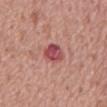| feature | finding |
|---|---|
| follow-up | imaged on a skin check; not biopsied |
| diameter | about 3 mm |
| image source | ~15 mm tile from a whole-body skin photo |
| body site | the back |
| image-analysis metrics | a lesion area of about 6 mm², an outline eccentricity of about 0.7 (0 = round, 1 = elongated), and a shape-asymmetry score of about 0.2 (0 = symmetric); a border-irregularity rating of about 2/10 and peripheral color asymmetry of about 1.5 |
| patient | male, roughly 70 years of age |
| lighting | white-light |